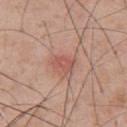Context: A male subject, in their mid-50s. The lesion is on the upper back. This is a white-light tile. Approximately 2.5 mm at its widest. A 15 mm close-up tile from a total-body photography series done for melanoma screening.